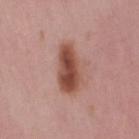Clinical impression:
Recorded during total-body skin imaging; not selected for excision or biopsy.
Acquisition and patient details:
Automated image analysis of the tile measured an area of roughly 12 mm² and a shape-asymmetry score of about 0.25 (0 = symmetric). And it measured a nevus-likeness score of about 100/100. The lesion is located on the leg. A 15 mm crop from a total-body photograph taken for skin-cancer surveillance. A female patient about 50 years old. The tile uses white-light illumination.